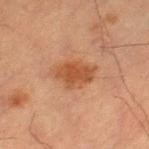Imaged during a routine full-body skin examination; the lesion was not biopsied and no histopathology is available.
Cropped from a whole-body photographic skin survey; the tile spans about 15 mm.
On the right thigh.
The recorded lesion diameter is about 5 mm.
The patient is a male about 65 years old.
Captured under cross-polarized illumination.
The lesion-visualizer software estimated a border-irregularity index near 2.5/10, internal color variation of about 2.5 on a 0–10 scale, and peripheral color asymmetry of about 0.5. And it measured a nevus-likeness score of about 65/100 and a detector confidence of about 100 out of 100 that the crop contains a lesion.